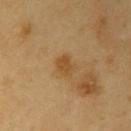Part of a total-body skin-imaging series; this lesion was reviewed on a skin check and was not flagged for biopsy. Captured under cross-polarized illumination. Automated image analysis of the tile measured a border-irregularity index near 2/10, a color-variation rating of about 1.5/10, and radial color variation of about 0.5. It also reported a nevus-likeness score of about 10/100 and lesion-presence confidence of about 100/100. The lesion's longest dimension is about 2.5 mm. This image is a 15 mm lesion crop taken from a total-body photograph. The lesion is located on the right upper arm. A male subject, aged approximately 60.